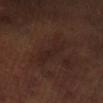Q: Was a biopsy performed?
A: no biopsy performed (imaged during a skin exam)
Q: What did automated image analysis measure?
A: a lesion color around L≈21 a*≈15 b*≈18 in CIELAB, roughly 4 lightness units darker than nearby skin, and a lesion-to-skin contrast of about 5.5 (normalized; higher = more distinct); an automated nevus-likeness rating near 0 out of 100 and a detector confidence of about 85 out of 100 that the crop contains a lesion
Q: What is the anatomic site?
A: the right lower leg
Q: What is the imaging modality?
A: 15 mm crop, total-body photography
Q: What are the patient's age and sex?
A: male, aged 48–52